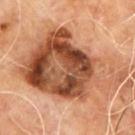Clinical impression:
Part of a total-body skin-imaging series; this lesion was reviewed on a skin check and was not flagged for biopsy.